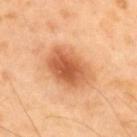| key | value |
|---|---|
| biopsy status | total-body-photography surveillance lesion; no biopsy |
| image | 15 mm crop, total-body photography |
| TBP lesion metrics | a footprint of about 17 mm², an eccentricity of roughly 0.7, and a shape-asymmetry score of about 0.15 (0 = symmetric); a mean CIELAB color near L≈60 a*≈28 b*≈41, roughly 14 lightness units darker than nearby skin, and a normalized border contrast of about 8.5; internal color variation of about 5 on a 0–10 scale and a peripheral color-asymmetry measure near 1.5; a nevus-likeness score of about 100/100 and a lesion-detection confidence of about 100/100 |
| anatomic site | the upper back |
| subject | male, aged 38–42 |
| lesion size | ~5.5 mm (longest diameter) |
| illumination | cross-polarized illumination |